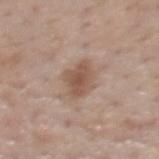Q: Was a biopsy performed?
A: imaged on a skin check; not biopsied
Q: Where on the body is the lesion?
A: the back
Q: What kind of image is this?
A: ~15 mm tile from a whole-body skin photo
Q: Automated lesion metrics?
A: a footprint of about 8 mm², an outline eccentricity of about 0.45 (0 = round, 1 = elongated), and a symmetry-axis asymmetry near 0.15; a lesion color around L≈53 a*≈18 b*≈27 in CIELAB and roughly 10 lightness units darker than nearby skin; a lesion-detection confidence of about 100/100
Q: Patient demographics?
A: male, aged 58 to 62
Q: Illumination type?
A: white-light illumination
Q: What is the lesion's diameter?
A: ~3 mm (longest diameter)